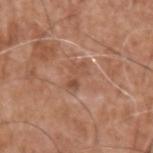This image is a 15 mm lesion crop taken from a total-body photograph. On the arm. A male patient, aged approximately 75.